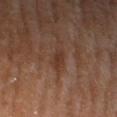notes — total-body-photography surveillance lesion; no biopsy | acquisition — ~15 mm tile from a whole-body skin photo | body site — the right thigh | patient — female, aged 58–62.